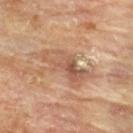| field | value |
|---|---|
| notes | catalogued during a skin exam; not biopsied |
| site | the upper back |
| patient | male, aged approximately 85 or older |
| tile lighting | cross-polarized |
| imaging modality | 15 mm crop, total-body photography |
| image-analysis metrics | a lesion–skin lightness drop of about 9 and a normalized border contrast of about 6.5 |
| diameter | ≈6.5 mm |